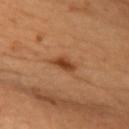Notes:
• follow-up · imaged on a skin check; not biopsied
• imaging modality · ~15 mm crop, total-body skin-cancer survey
• tile lighting · cross-polarized
• size · ≈3 mm
• automated lesion analysis · a lesion color around L≈37 a*≈21 b*≈32 in CIELAB and a lesion-to-skin contrast of about 8.5 (normalized; higher = more distinct); radial color variation of about 0.5; a classifier nevus-likeness of about 95/100
• anatomic site · the front of the torso
• subject · female, aged 58 to 62